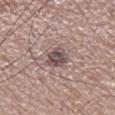Case summary:
- biopsy status: total-body-photography surveillance lesion; no biopsy
- patient: male, aged 63–67
- image: ~15 mm tile from a whole-body skin photo
- illumination: white-light
- location: the right lower leg
- diameter: ≈3 mm